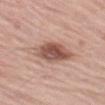follow-up = catalogued during a skin exam; not biopsied | illumination = white-light illumination | imaging modality = total-body-photography crop, ~15 mm field of view | location = the left thigh | lesion diameter = about 6.5 mm | patient = male, in their 80s.